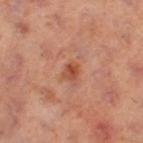| key | value |
|---|---|
| biopsy status | catalogued during a skin exam; not biopsied |
| automated metrics | an area of roughly 3 mm² and a symmetry-axis asymmetry near 0.25; a lesion-to-skin contrast of about 8 (normalized; higher = more distinct) |
| patient | female, aged 38–42 |
| anatomic site | the left thigh |
| lesion diameter | ~2 mm (longest diameter) |
| image source | total-body-photography crop, ~15 mm field of view |